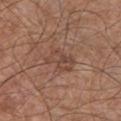workup=total-body-photography surveillance lesion; no biopsy | body site=the chest | image=~15 mm crop, total-body skin-cancer survey | subject=male, approximately 65 years of age | illumination=white-light | lesion size=≈4 mm.